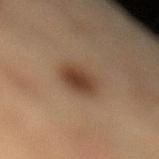biopsy status — no biopsy performed (imaged during a skin exam) | subject — male, approximately 85 years of age or older | image source — ~15 mm tile from a whole-body skin photo | TBP lesion metrics — an automated nevus-likeness rating near 100 out of 100 and a detector confidence of about 100 out of 100 that the crop contains a lesion | location — the left lower leg | lesion size — ~4 mm (longest diameter).